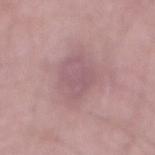The lesion was tiled from a total-body skin photograph and was not biopsied. A close-up tile cropped from a whole-body skin photograph, about 15 mm across. The tile uses white-light illumination. The lesion is on the abdomen. A male subject in their mid-70s. Measured at roughly 5 mm in maximum diameter. Automated image analysis of the tile measured an area of roughly 14 mm², a shape eccentricity near 0.65, and two-axis asymmetry of about 0.2. And it measured border irregularity of about 2.5 on a 0–10 scale, internal color variation of about 2 on a 0–10 scale, and peripheral color asymmetry of about 0.5.